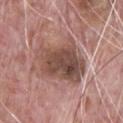Q: How large is the lesion?
A: ≈6.5 mm
Q: What is the imaging modality?
A: ~15 mm crop, total-body skin-cancer survey
Q: Lesion location?
A: the chest
Q: Automated lesion metrics?
A: a mean CIELAB color near L≈48 a*≈19 b*≈24, roughly 12 lightness units darker than nearby skin, and a normalized border contrast of about 9; a border-irregularity index near 3.5/10, a within-lesion color-variation index near 6.5/10, and peripheral color asymmetry of about 2; an automated nevus-likeness rating near 0 out of 100 and a detector confidence of about 100 out of 100 that the crop contains a lesion
Q: Who is the patient?
A: male, aged 68–72